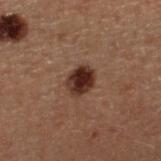The lesion was photographed on a routine skin check and not biopsied; there is no pathology result. The lesion is on the right lower leg. Imaged with cross-polarized lighting. An algorithmic analysis of the crop reported a lesion color around L≈22 a*≈16 b*≈19 in CIELAB, roughly 12 lightness units darker than nearby skin, and a normalized lesion–skin contrast near 13. It also reported a border-irregularity index near 1.5/10 and internal color variation of about 4.5 on a 0–10 scale. This image is a 15 mm lesion crop taken from a total-body photograph. The subject is a male aged approximately 60. The recorded lesion diameter is about 3 mm.